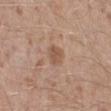biopsy status — imaged on a skin check; not biopsied
subject — male, aged around 45
tile lighting — white-light
site — the right lower leg
size — about 2.5 mm
image source — ~15 mm tile from a whole-body skin photo